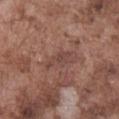Impression:
This lesion was catalogued during total-body skin photography and was not selected for biopsy.
Image and clinical context:
A region of skin cropped from a whole-body photographic capture, roughly 15 mm wide. This is a white-light tile. The lesion's longest dimension is about 3.5 mm. A male subject, approximately 75 years of age. From the abdomen.